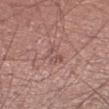| key | value |
|---|---|
| biopsy status | imaged on a skin check; not biopsied |
| subject | male, aged 43 to 47 |
| image source | ~15 mm tile from a whole-body skin photo |
| lighting | white-light illumination |
| automated metrics | an outline eccentricity of about 0.9 (0 = round, 1 = elongated) and a symmetry-axis asymmetry near 0.35; a nevus-likeness score of about 0/100 |
| size | ≈3.5 mm |
| body site | the left lower leg |